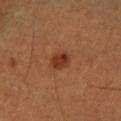biopsy status: imaged on a skin check; not biopsied | lesion diameter: ≈2.5 mm | image source: ~15 mm tile from a whole-body skin photo | image-analysis metrics: two-axis asymmetry of about 0.2; a mean CIELAB color near L≈36 a*≈25 b*≈33 and a lesion-to-skin contrast of about 8.5 (normalized; higher = more distinct); border irregularity of about 1.5 on a 0–10 scale; a lesion-detection confidence of about 100/100 | location: the left lower leg | subject: male, approximately 50 years of age | lighting: cross-polarized.